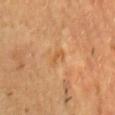lighting — cross-polarized illumination | imaging modality — ~15 mm tile from a whole-body skin photo | patient — male, aged approximately 60 | automated lesion analysis — an area of roughly 2 mm² and a shape-asymmetry score of about 0.4 (0 = symmetric); a lesion color around L≈52 a*≈22 b*≈40 in CIELAB and a lesion-to-skin contrast of about 5.5 (normalized; higher = more distinct); border irregularity of about 4 on a 0–10 scale and a peripheral color-asymmetry measure near 0 | site — the chest | diameter — about 2 mm.